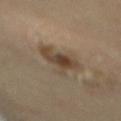Notes:
– workup — no biopsy performed (imaged during a skin exam)
– diameter — about 5 mm
– anatomic site — the mid back
– illumination — cross-polarized illumination
– image — ~15 mm tile from a whole-body skin photo
– patient — female, about 70 years old